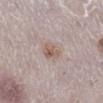  biopsy_status: not biopsied; imaged during a skin examination
  image:
    source: total-body photography crop
    field_of_view_mm: 15
  site: right lower leg
  lesion_size:
    long_diameter_mm_approx: 2.5
  patient:
    sex: female
    age_approx: 50
  automated_metrics:
    cielab_L: 58
    cielab_a: 15
    cielab_b: 23
    vs_skin_darker_L: 8.0
    vs_skin_contrast_norm: 6.5
    border_irregularity_0_10: 2.0
    color_variation_0_10: 4.5